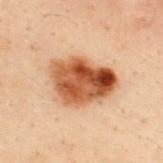Imaged during a routine full-body skin examination; the lesion was not biopsied and no histopathology is available.
On the upper back.
Longest diameter approximately 6.5 mm.
A male patient aged 28–32.
Cropped from a total-body skin-imaging series; the visible field is about 15 mm.
Imaged with cross-polarized lighting.